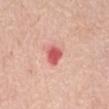Clinical impression: The lesion was tiled from a total-body skin photograph and was not biopsied. Clinical summary: Captured under white-light illumination. The lesion's longest dimension is about 2.5 mm. This image is a 15 mm lesion crop taken from a total-body photograph. The lesion is on the abdomen. The subject is a female aged 73 to 77.